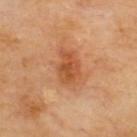This lesion was catalogued during total-body skin photography and was not selected for biopsy. Imaged with cross-polarized lighting. From the upper back. The lesion's longest dimension is about 4.5 mm. A close-up tile cropped from a whole-body skin photograph, about 15 mm across. The lesion-visualizer software estimated a footprint of about 9.5 mm² and an eccentricity of roughly 0.8. The software also gave a border-irregularity index near 2.5/10, internal color variation of about 4 on a 0–10 scale, and a peripheral color-asymmetry measure near 1. The analysis additionally found lesion-presence confidence of about 100/100.